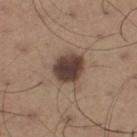<case>
<biopsy_status>not biopsied; imaged during a skin examination</biopsy_status>
<site>left thigh</site>
<image>
  <source>total-body photography crop</source>
  <field_of_view_mm>15</field_of_view_mm>
</image>
<patient>
  <sex>male</sex>
  <age_approx>65</age_approx>
</patient>
<automated_metrics>
  <area_mm2_approx>12.0</area_mm2_approx>
  <eccentricity>0.35</eccentricity>
  <shape_asymmetry>0.25</shape_asymmetry>
</automated_metrics>
<lesion_size>
  <long_diameter_mm_approx>4.0</long_diameter_mm_approx>
</lesion_size>
<lighting>white-light</lighting>
</case>